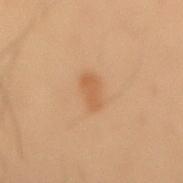No biopsy was performed on this lesion — it was imaged during a full skin examination and was not determined to be concerning. From the lower back. A male subject aged 53 to 57. Automated image analysis of the tile measured an area of roughly 4.5 mm², an outline eccentricity of about 0.9 (0 = round, 1 = elongated), and two-axis asymmetry of about 0.3. The software also gave a border-irregularity index near 3/10, a within-lesion color-variation index near 1/10, and a peripheral color-asymmetry measure near 0.5. The lesion's longest dimension is about 3.5 mm. A 15 mm close-up tile from a total-body photography series done for melanoma screening. This is a cross-polarized tile.